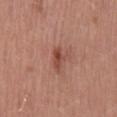Q: Is there a histopathology result?
A: total-body-photography surveillance lesion; no biopsy
Q: Illumination type?
A: white-light illumination
Q: Patient demographics?
A: female, aged approximately 35
Q: What did automated image analysis measure?
A: an area of roughly 3.5 mm², a shape eccentricity near 0.85, and two-axis asymmetry of about 0.3; a within-lesion color-variation index near 3.5/10 and a peripheral color-asymmetry measure near 1.5
Q: Where on the body is the lesion?
A: the back
Q: What is the imaging modality?
A: ~15 mm crop, total-body skin-cancer survey